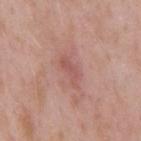Captured during whole-body skin photography for melanoma surveillance; the lesion was not biopsied.
About 4 mm across.
The lesion is located on the mid back.
Captured under white-light illumination.
The subject is a male in their mid-50s.
The lesion-visualizer software estimated a lesion area of about 4.5 mm², an outline eccentricity of about 0.9 (0 = round, 1 = elongated), and a shape-asymmetry score of about 0.4 (0 = symmetric). And it measured border irregularity of about 4 on a 0–10 scale and internal color variation of about 2 on a 0–10 scale.
A 15 mm crop from a total-body photograph taken for skin-cancer surveillance.